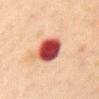Imaged during a routine full-body skin examination; the lesion was not biopsied and no histopathology is available. This is a cross-polarized tile. On the chest. A roughly 15 mm field-of-view crop from a total-body skin photograph. An algorithmic analysis of the crop reported an area of roughly 11 mm² and an outline eccentricity of about 0.45 (0 = round, 1 = elongated). The software also gave border irregularity of about 1 on a 0–10 scale, a within-lesion color-variation index near 7/10, and radial color variation of about 2. The software also gave a classifier nevus-likeness of about 0/100 and a lesion-detection confidence of about 100/100. The subject is a female approximately 40 years of age. The recorded lesion diameter is about 4 mm.